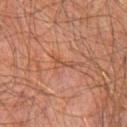  biopsy_status: not biopsied; imaged during a skin examination
  patient:
    sex: male
    age_approx: 60
  site: chest
  image:
    source: total-body photography crop
    field_of_view_mm: 15